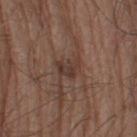The lesion was photographed on a routine skin check and not biopsied; there is no pathology result.
The lesion is on the left thigh.
A lesion tile, about 15 mm wide, cut from a 3D total-body photograph.
Captured under white-light illumination.
A male subject, about 75 years old.
The recorded lesion diameter is about 3 mm.